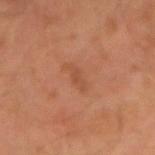biopsy_status: not biopsied; imaged during a skin examination
site: right upper arm
image:
  source: total-body photography crop
  field_of_view_mm: 15
lighting: cross-polarized
patient:
  sex: male
  age_approx: 60
lesion_size:
  long_diameter_mm_approx: 3.0
automated_metrics:
  cielab_L: 45
  cielab_a: 23
  cielab_b: 31
  vs_skin_darker_L: 6.0
  border_irregularity_0_10: 4.0
  color_variation_0_10: 0.0
  peripheral_color_asymmetry: 0.0
  nevus_likeness_0_100: 0
  lesion_detection_confidence_0_100: 100